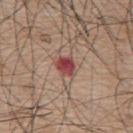biopsy_status: not biopsied; imaged during a skin examination
lesion_size:
  long_diameter_mm_approx: 3.0
site: mid back
automated_metrics:
  area_mm2_approx: 5.0
  eccentricity: 0.65
  cielab_L: 46
  cielab_a: 29
  cielab_b: 23
  vs_skin_darker_L: 14.0
  vs_skin_contrast_norm: 10.5
  border_irregularity_0_10: 2.0
  peripheral_color_asymmetry: 1.5
  nevus_likeness_0_100: 0
  lesion_detection_confidence_0_100: 100
lighting: white-light
image:
  source: total-body photography crop
  field_of_view_mm: 15
patient:
  sex: male
  age_approx: 75